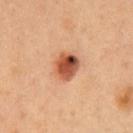Q: Was a biopsy performed?
A: imaged on a skin check; not biopsied
Q: Lesion size?
A: ≈3.5 mm
Q: What did automated image analysis measure?
A: a lesion area of about 8 mm², a shape eccentricity near 0.5, and a shape-asymmetry score of about 0.2 (0 = symmetric); about 17 CIELAB-L* units darker than the surrounding skin
Q: How was the tile lit?
A: cross-polarized illumination
Q: Who is the patient?
A: male, aged 48–52
Q: What is the imaging modality?
A: ~15 mm tile from a whole-body skin photo
Q: Lesion location?
A: the chest